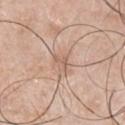acquisition: total-body-photography crop, ~15 mm field of view | patient: male, aged 48 to 52 | image-analysis metrics: a lesion area of about 2.5 mm², an eccentricity of roughly 0.85, and two-axis asymmetry of about 0.55; radial color variation of about 0 | lesion diameter: ~2.5 mm (longest diameter) | tile lighting: white-light | body site: the front of the torso.